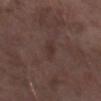{"lesion_size": {"long_diameter_mm_approx": 2.5}, "lighting": "white-light", "site": "right forearm", "image": {"source": "total-body photography crop", "field_of_view_mm": 15}, "automated_metrics": {"eccentricity": 0.85, "nevus_likeness_0_100": 0, "lesion_detection_confidence_0_100": 100}, "patient": {"sex": "male", "age_approx": 75}}